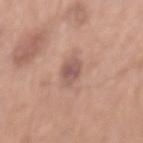{
  "biopsy_status": "not biopsied; imaged during a skin examination",
  "site": "mid back",
  "image": {
    "source": "total-body photography crop",
    "field_of_view_mm": 15
  },
  "lighting": "white-light",
  "automated_metrics": {
    "area_mm2_approx": 4.5,
    "eccentricity": 0.8,
    "shape_asymmetry": 0.2,
    "border_irregularity_0_10": 2.0,
    "color_variation_0_10": 2.0,
    "peripheral_color_asymmetry": 0.5
  },
  "patient": {
    "sex": "male",
    "age_approx": 70
  }
}